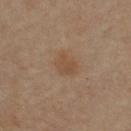Part of a total-body skin-imaging series; this lesion was reviewed on a skin check and was not flagged for biopsy.
Located on the left upper arm.
The total-body-photography lesion software estimated a lesion color around L≈48 a*≈17 b*≈30 in CIELAB, roughly 6 lightness units darker than nearby skin, and a lesion-to-skin contrast of about 6 (normalized; higher = more distinct). The software also gave an automated nevus-likeness rating near 50 out of 100 and lesion-presence confidence of about 100/100.
A male subject, aged 63 to 67.
Cropped from a whole-body photographic skin survey; the tile spans about 15 mm.
The recorded lesion diameter is about 3 mm.